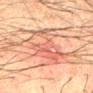notes: catalogued during a skin exam; not biopsied.